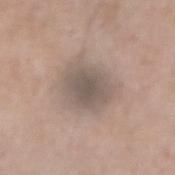Clinical impression:
This lesion was catalogued during total-body skin photography and was not selected for biopsy.